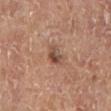notes: catalogued during a skin exam; not biopsied
anatomic site: the left lower leg
illumination: cross-polarized
subject: male, aged approximately 65
diameter: about 2.5 mm
TBP lesion metrics: a lesion area of about 4.5 mm², an outline eccentricity of about 0.45 (0 = round, 1 = elongated), and a shape-asymmetry score of about 0.3 (0 = symmetric); a nevus-likeness score of about 40/100 and a lesion-detection confidence of about 100/100
acquisition: ~15 mm crop, total-body skin-cancer survey